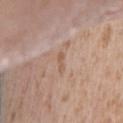{"biopsy_status": "not biopsied; imaged during a skin examination", "site": "abdomen", "lighting": "white-light", "patient": {"sex": "male", "age_approx": 45}, "lesion_size": {"long_diameter_mm_approx": 2.5}, "automated_metrics": {"area_mm2_approx": 2.0, "eccentricity": 0.95, "shape_asymmetry": 0.3, "vs_skin_contrast_norm": 5.5}, "image": {"source": "total-body photography crop", "field_of_view_mm": 15}}